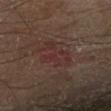Clinical impression: Part of a total-body skin-imaging series; this lesion was reviewed on a skin check and was not flagged for biopsy. Clinical summary: Cropped from a whole-body photographic skin survey; the tile spans about 15 mm. A male patient, aged 68–72. The tile uses cross-polarized illumination. The lesion is on the leg. Measured at roughly 5 mm in maximum diameter.